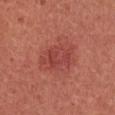Clinical impression:
Recorded during total-body skin imaging; not selected for excision or biopsy.
Image and clinical context:
Automated tile analysis of the lesion measured an automated nevus-likeness rating near 50 out of 100 and lesion-presence confidence of about 100/100. Imaged with white-light lighting. The recorded lesion diameter is about 5 mm. A region of skin cropped from a whole-body photographic capture, roughly 15 mm wide. A female patient, in their mid- to late 30s. The lesion is on the upper back.